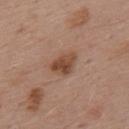{"biopsy_status": "not biopsied; imaged during a skin examination", "lesion_size": {"long_diameter_mm_approx": 3.5}, "lighting": "white-light", "site": "upper back", "patient": {"sex": "male", "age_approx": 55}, "image": {"source": "total-body photography crop", "field_of_view_mm": 15}}